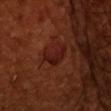Q: How was the tile lit?
A: cross-polarized
Q: How large is the lesion?
A: about 3 mm
Q: Who is the patient?
A: male, roughly 50 years of age
Q: What kind of image is this?
A: 15 mm crop, total-body photography
Q: Where on the body is the lesion?
A: the head or neck
Q: What did automated image analysis measure?
A: a nevus-likeness score of about 95/100